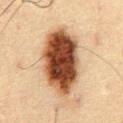The lesion was tiled from a total-body skin photograph and was not biopsied.
The lesion is located on the abdomen.
Longest diameter approximately 8.5 mm.
Cropped from a total-body skin-imaging series; the visible field is about 15 mm.
The total-body-photography lesion software estimated about 21 CIELAB-L* units darker than the surrounding skin and a normalized lesion–skin contrast near 16. The software also gave a classifier nevus-likeness of about 100/100 and a detector confidence of about 100 out of 100 that the crop contains a lesion.
A male subject, aged approximately 60.
Imaged with cross-polarized lighting.